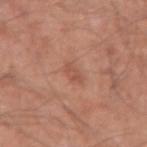{"biopsy_status": "not biopsied; imaged during a skin examination", "image": {"source": "total-body photography crop", "field_of_view_mm": 15}, "site": "right upper arm", "patient": {"sex": "male", "age_approx": 60}}